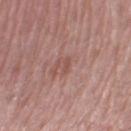Clinical impression: Part of a total-body skin-imaging series; this lesion was reviewed on a skin check and was not flagged for biopsy. Image and clinical context: A male patient, roughly 60 years of age. Captured under white-light illumination. Longest diameter approximately 2 mm. Cropped from a whole-body photographic skin survey; the tile spans about 15 mm. On the right thigh.